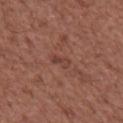  biopsy_status: not biopsied; imaged during a skin examination
  lighting: white-light
  automated_metrics:
    area_mm2_approx: 2.5
    eccentricity: 0.9
    shape_asymmetry: 0.4
  image:
    source: total-body photography crop
    field_of_view_mm: 15
  lesion_size:
    long_diameter_mm_approx: 2.5
  patient:
    sex: male
    age_approx: 75
  site: chest